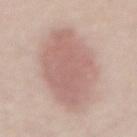subject: male, in their 60s
tile lighting: white-light
site: the abdomen
acquisition: 15 mm crop, total-body photography